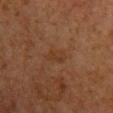Assessment: The lesion was photographed on a routine skin check and not biopsied; there is no pathology result. Image and clinical context: Located on the chest. The patient is a female approximately 60 years of age. Automated image analysis of the tile measured a lesion area of about 4 mm², a shape eccentricity near 0.8, and a symmetry-axis asymmetry near 0.25. The software also gave an average lesion color of about L≈29 a*≈17 b*≈26 (CIELAB) and a lesion–skin lightness drop of about 4. The software also gave a nevus-likeness score of about 0/100 and a lesion-detection confidence of about 100/100. The recorded lesion diameter is about 3 mm. A region of skin cropped from a whole-body photographic capture, roughly 15 mm wide. Imaged with cross-polarized lighting.